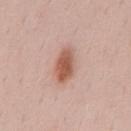No biopsy was performed on this lesion — it was imaged during a full skin examination and was not determined to be concerning. The lesion-visualizer software estimated border irregularity of about 2 on a 0–10 scale, internal color variation of about 4 on a 0–10 scale, and a peripheral color-asymmetry measure near 1. A region of skin cropped from a whole-body photographic capture, roughly 15 mm wide. A male subject aged approximately 50. The lesion's longest dimension is about 4.5 mm. From the mid back.